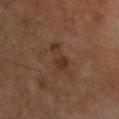Findings:
- biopsy status — no biopsy performed (imaged during a skin exam)
- image source — total-body-photography crop, ~15 mm field of view
- size — ≈4 mm
- location — the chest
- patient — male, aged 53 to 57
- image-analysis metrics — an outline eccentricity of about 0.85 (0 = round, 1 = elongated) and two-axis asymmetry of about 0.35; roughly 6 lightness units darker than nearby skin and a normalized border contrast of about 6.5; a nevus-likeness score of about 0/100 and lesion-presence confidence of about 100/100